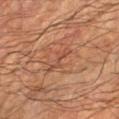notes — imaged on a skin check; not biopsied
tile lighting — cross-polarized
site — the left forearm
TBP lesion metrics — a lesion area of about 3.5 mm² and two-axis asymmetry of about 0.6; border irregularity of about 8.5 on a 0–10 scale, internal color variation of about 0 on a 0–10 scale, and peripheral color asymmetry of about 0
lesion diameter — ≈3.5 mm
image source — ~15 mm crop, total-body skin-cancer survey
subject — male, in their 60s